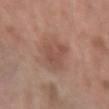Captured during whole-body skin photography for melanoma surveillance; the lesion was not biopsied. A female subject roughly 60 years of age. Located on the left forearm. Cropped from a whole-body photographic skin survey; the tile spans about 15 mm.